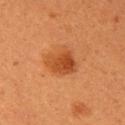follow-up: imaged on a skin check; not biopsied
diameter: about 3.5 mm
patient: female, about 30 years old
location: the right upper arm
image: 15 mm crop, total-body photography